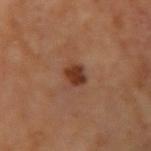notes: total-body-photography surveillance lesion; no biopsy
image source: ~15 mm crop, total-body skin-cancer survey
subject: male, about 65 years old
location: the left arm